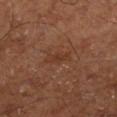This lesion was catalogued during total-body skin photography and was not selected for biopsy.
The lesion is on the right lower leg.
The subject is aged around 65.
Cropped from a total-body skin-imaging series; the visible field is about 15 mm.